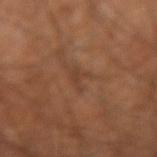No biopsy was performed on this lesion — it was imaged during a full skin examination and was not determined to be concerning. A male patient approximately 45 years of age. The recorded lesion diameter is about 3 mm. From the right forearm. This image is a 15 mm lesion crop taken from a total-body photograph. The total-body-photography lesion software estimated a footprint of about 3 mm², a shape eccentricity near 0.85, and two-axis asymmetry of about 0.5. The analysis additionally found a normalized border contrast of about 5. The analysis additionally found a border-irregularity index near 6/10, a color-variation rating of about 0/10, and a peripheral color-asymmetry measure near 0. The analysis additionally found lesion-presence confidence of about 65/100.